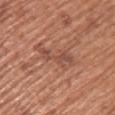{"biopsy_status": "not biopsied; imaged during a skin examination", "patient": {"sex": "female", "age_approx": 60}, "image": {"source": "total-body photography crop", "field_of_view_mm": 15}, "site": "upper back"}